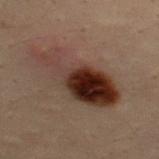Q: Is there a histopathology result?
A: imaged on a skin check; not biopsied
Q: Lesion location?
A: the upper back
Q: What are the patient's age and sex?
A: male, roughly 30 years of age
Q: Illumination type?
A: cross-polarized
Q: What kind of image is this?
A: 15 mm crop, total-body photography
Q: What is the lesion's diameter?
A: ≈9 mm